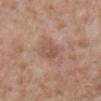Assessment:
Part of a total-body skin-imaging series; this lesion was reviewed on a skin check and was not flagged for biopsy.
Background:
The recorded lesion diameter is about 3 mm. A 15 mm close-up tile from a total-body photography series done for melanoma screening. The lesion is on the chest. Captured under white-light illumination. The total-body-photography lesion software estimated a lesion area of about 5.5 mm², an outline eccentricity of about 0.75 (0 = round, 1 = elongated), and a symmetry-axis asymmetry near 0.15. It also reported roughly 8 lightness units darker than nearby skin and a normalized lesion–skin contrast near 5.5. The analysis additionally found a border-irregularity index near 1.5/10, internal color variation of about 3 on a 0–10 scale, and peripheral color asymmetry of about 1. The patient is a male approximately 60 years of age.